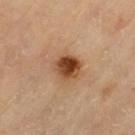Captured during whole-body skin photography for melanoma surveillance; the lesion was not biopsied.
The total-body-photography lesion software estimated a lesion area of about 8 mm², a shape eccentricity near 0.4, and two-axis asymmetry of about 0.15. The analysis additionally found internal color variation of about 8.5 on a 0–10 scale and a peripheral color-asymmetry measure near 3.
The lesion's longest dimension is about 3.5 mm.
A 15 mm close-up tile from a total-body photography series done for melanoma screening.
Located on the left thigh.
Imaged with cross-polarized lighting.
A female patient, aged approximately 70.